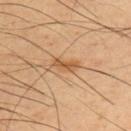The lesion was tiled from a total-body skin photograph and was not biopsied. An algorithmic analysis of the crop reported an average lesion color of about L≈54 a*≈20 b*≈38 (CIELAB) and a lesion–skin lightness drop of about 10. The analysis additionally found a border-irregularity rating of about 4/10 and a peripheral color-asymmetry measure near 0.5. The software also gave a detector confidence of about 100 out of 100 that the crop contains a lesion. The subject is a male aged around 60. A 15 mm crop from a total-body photograph taken for skin-cancer surveillance. Approximately 3 mm at its widest. From the back.